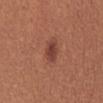Recorded during total-body skin imaging; not selected for excision or biopsy. From the abdomen. Cropped from a total-body skin-imaging series; the visible field is about 15 mm. The patient is a female aged 23–27.